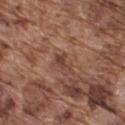This lesion was catalogued during total-body skin photography and was not selected for biopsy.
A 15 mm crop from a total-body photograph taken for skin-cancer surveillance.
The lesion is on the upper back.
A male patient aged 73 to 77.